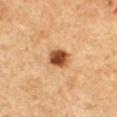follow-up = total-body-photography surveillance lesion; no biopsy.